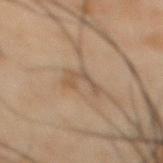- workup · total-body-photography surveillance lesion; no biopsy
- patient · male, aged approximately 50
- tile lighting · cross-polarized
- location · the abdomen
- automated lesion analysis · an average lesion color of about L≈40 a*≈12 b*≈24 (CIELAB); a border-irregularity rating of about 8/10 and radial color variation of about 0
- image · total-body-photography crop, ~15 mm field of view
- size · ~3 mm (longest diameter)